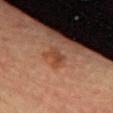Imaged during a routine full-body skin examination; the lesion was not biopsied and no histopathology is available. A 15 mm close-up tile from a total-body photography series done for melanoma screening. The tile uses cross-polarized illumination. A female subject, aged approximately 65. The lesion-visualizer software estimated an area of roughly 3.5 mm² and a shape eccentricity near 0.8. Approximately 2.5 mm at its widest. The lesion is on the chest.